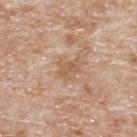Clinical impression: No biopsy was performed on this lesion — it was imaged during a full skin examination and was not determined to be concerning. Context: The lesion-visualizer software estimated a mean CIELAB color near L≈58 a*≈19 b*≈33 and a lesion–skin lightness drop of about 8. The software also gave a border-irregularity index near 4/10, internal color variation of about 2.5 on a 0–10 scale, and peripheral color asymmetry of about 1. This is a white-light tile. A male patient, roughly 80 years of age. A roughly 15 mm field-of-view crop from a total-body skin photograph. Approximately 2.5 mm at its widest. From the upper back.